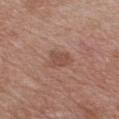biopsy status=catalogued during a skin exam; not biopsied
location=the chest
imaging modality=total-body-photography crop, ~15 mm field of view
size=~3 mm (longest diameter)
subject=male, approximately 65 years of age